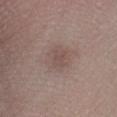workup=total-body-photography surveillance lesion; no biopsy | body site=the left lower leg | lesion size=about 5 mm | subject=male, aged approximately 45 | lighting=white-light | imaging modality=~15 mm crop, total-body skin-cancer survey.